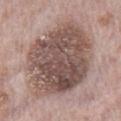<record>
<biopsy_status>not biopsied; imaged during a skin examination</biopsy_status>
<image>
  <source>total-body photography crop</source>
  <field_of_view_mm>15</field_of_view_mm>
</image>
<lighting>white-light</lighting>
<site>chest</site>
<lesion_size>
  <long_diameter_mm_approx>11.0</long_diameter_mm_approx>
</lesion_size>
<patient>
  <sex>male</sex>
  <age_approx>70</age_approx>
</patient>
</record>